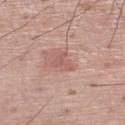The lesion is located on the left lower leg.
The subject is a male aged around 70.
Measured at roughly 2.5 mm in maximum diameter.
A region of skin cropped from a whole-body photographic capture, roughly 15 mm wide.
This is a white-light tile.